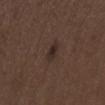A male patient about 70 years old.
Located on the mid back.
Automated image analysis of the tile measured an area of roughly 3.5 mm². The software also gave a lesion-to-skin contrast of about 8 (normalized; higher = more distinct). The analysis additionally found a nevus-likeness score of about 90/100.
Approximately 3 mm at its widest.
A lesion tile, about 15 mm wide, cut from a 3D total-body photograph.